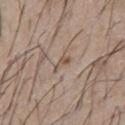Part of a total-body skin-imaging series; this lesion was reviewed on a skin check and was not flagged for biopsy. This image is a 15 mm lesion crop taken from a total-body photograph. The lesion's longest dimension is about 2.5 mm. On the chest. The patient is a male in their mid-60s.